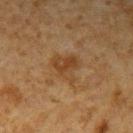{"biopsy_status": "not biopsied; imaged during a skin examination", "image": {"source": "total-body photography crop", "field_of_view_mm": 15}, "site": "left upper arm", "patient": {"sex": "male", "age_approx": 60}, "automated_metrics": {"cielab_L": 35, "cielab_a": 16, "cielab_b": 30, "vs_skin_darker_L": 6.0, "vs_skin_contrast_norm": 6.5, "border_irregularity_0_10": 5.0, "color_variation_0_10": 3.5, "nevus_likeness_0_100": 0, "lesion_detection_confidence_0_100": 100}, "lighting": "cross-polarized", "lesion_size": {"long_diameter_mm_approx": 3.5}}